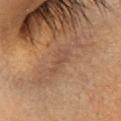| field | value |
|---|---|
| notes | imaged on a skin check; not biopsied |
| body site | the head or neck |
| patient | female, aged approximately 30 |
| tile lighting | cross-polarized illumination |
| acquisition | total-body-photography crop, ~15 mm field of view |